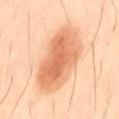Assessment: This lesion was catalogued during total-body skin photography and was not selected for biopsy. Image and clinical context: The patient is a male roughly 40 years of age. The total-body-photography lesion software estimated an area of roughly 31 mm², a shape eccentricity near 0.8, and a shape-asymmetry score of about 0.15 (0 = symmetric). It also reported border irregularity of about 2 on a 0–10 scale, a within-lesion color-variation index near 5.5/10, and a peripheral color-asymmetry measure near 1.5. The software also gave a nevus-likeness score of about 100/100 and a lesion-detection confidence of about 100/100. About 8 mm across. From the lower back. Imaged with cross-polarized lighting. This image is a 15 mm lesion crop taken from a total-body photograph.